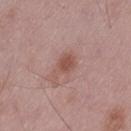Recorded during total-body skin imaging; not selected for excision or biopsy.
Captured under white-light illumination.
The lesion is located on the left thigh.
Approximately 3.5 mm at its widest.
A close-up tile cropped from a whole-body skin photograph, about 15 mm across.
A male patient aged 48–52.
The total-body-photography lesion software estimated a lesion area of about 5.5 mm² and a shape eccentricity near 0.8. The software also gave a border-irregularity rating of about 4/10 and a peripheral color-asymmetry measure near 0.5. The software also gave an automated nevus-likeness rating near 50 out of 100 and a lesion-detection confidence of about 100/100.